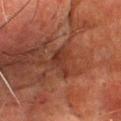The lesion was tiled from a total-body skin photograph and was not biopsied.
On the chest.
A male subject aged 63–67.
Imaged with cross-polarized lighting.
A 15 mm crop from a total-body photograph taken for skin-cancer surveillance.
The recorded lesion diameter is about 3.5 mm.